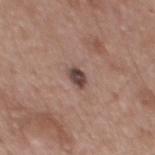Impression:
The lesion was photographed on a routine skin check and not biopsied; there is no pathology result.
Image and clinical context:
Automated image analysis of the tile measured a mean CIELAB color near L≈44 a*≈17 b*≈20, roughly 14 lightness units darker than nearby skin, and a normalized lesion–skin contrast near 11. And it measured a border-irregularity rating of about 1.5/10, internal color variation of about 3 on a 0–10 scale, and radial color variation of about 1. A region of skin cropped from a whole-body photographic capture, roughly 15 mm wide. About 2.5 mm across. Located on the mid back. A male subject, approximately 55 years of age. This is a white-light tile.